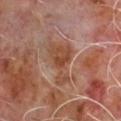Findings:
* notes — imaged on a skin check; not biopsied
* body site — the chest
* lesion size — about 5.5 mm
* subject — male, about 65 years old
* automated metrics — a lesion area of about 11 mm², an outline eccentricity of about 0.85 (0 = round, 1 = elongated), and two-axis asymmetry of about 0.6; a mean CIELAB color near L≈45 a*≈21 b*≈30, a lesion–skin lightness drop of about 7, and a normalized border contrast of about 7; border irregularity of about 7 on a 0–10 scale, internal color variation of about 3.5 on a 0–10 scale, and peripheral color asymmetry of about 1; a classifier nevus-likeness of about 0/100 and a lesion-detection confidence of about 95/100
* acquisition — ~15 mm tile from a whole-body skin photo
* lighting — cross-polarized